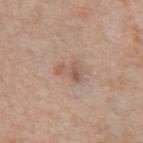notes=total-body-photography surveillance lesion; no biopsy | patient=male, approximately 70 years of age | imaging modality=~15 mm crop, total-body skin-cancer survey | site=the abdomen.